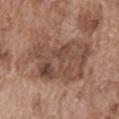notes: imaged on a skin check; not biopsied
TBP lesion metrics: a shape eccentricity near 0.7 and a shape-asymmetry score of about 0.3 (0 = symmetric); a detector confidence of about 100 out of 100 that the crop contains a lesion
subject: male, about 75 years old
acquisition: total-body-photography crop, ~15 mm field of view
diameter: ~8.5 mm (longest diameter)
location: the abdomen
tile lighting: white-light